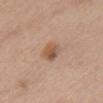Notes:
* biopsy status — no biopsy performed (imaged during a skin exam)
* patient — female, aged 48 to 52
* TBP lesion metrics — a lesion-to-skin contrast of about 7.5 (normalized; higher = more distinct); a border-irregularity rating of about 1/10, a within-lesion color-variation index near 5.5/10, and a peripheral color-asymmetry measure near 2; an automated nevus-likeness rating near 80 out of 100 and a detector confidence of about 100 out of 100 that the crop contains a lesion
* anatomic site — the chest
* image — ~15 mm crop, total-body skin-cancer survey
* illumination — white-light illumination